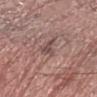Recorded during total-body skin imaging; not selected for excision or biopsy.
A roughly 15 mm field-of-view crop from a total-body skin photograph.
A male patient in their 70s.
The lesion is located on the left forearm.
The lesion's longest dimension is about 3 mm.
This is a white-light tile.